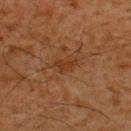{
  "biopsy_status": "not biopsied; imaged during a skin examination",
  "image": {
    "source": "total-body photography crop",
    "field_of_view_mm": 15
  },
  "site": "upper back",
  "patient": {
    "sex": "male",
    "age_approx": 60
  },
  "lighting": "cross-polarized",
  "lesion_size": {
    "long_diameter_mm_approx": 2.5
  }
}